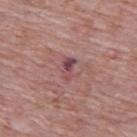workup = no biopsy performed (imaged during a skin exam)
image = total-body-photography crop, ~15 mm field of view
TBP lesion metrics = a lesion color around L≈47 a*≈24 b*≈17 in CIELAB, a lesion–skin lightness drop of about 9, and a normalized lesion–skin contrast near 8; border irregularity of about 3.5 on a 0–10 scale, a color-variation rating of about 7.5/10, and radial color variation of about 3; a nevus-likeness score of about 0/100
size = about 3 mm
body site = the upper back
tile lighting = white-light
subject = male, approximately 65 years of age